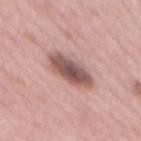The lesion was tiled from a total-body skin photograph and was not biopsied.
Cropped from a total-body skin-imaging series; the visible field is about 15 mm.
The lesion is located on the mid back.
A female patient, aged 63–67.
The lesion-visualizer software estimated a lesion area of about 12 mm², an eccentricity of roughly 0.9, and two-axis asymmetry of about 0.15. It also reported a lesion color around L≈53 a*≈21 b*≈21 in CIELAB, a lesion–skin lightness drop of about 16, and a normalized lesion–skin contrast near 10. And it measured a border-irregularity index near 2.5/10, a within-lesion color-variation index near 5.5/10, and peripheral color asymmetry of about 1.5. The analysis additionally found an automated nevus-likeness rating near 90 out of 100 and a lesion-detection confidence of about 100/100.
Imaged with white-light lighting.
The lesion's longest dimension is about 6 mm.